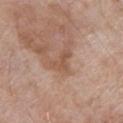Image and clinical context: A male patient in their 80s. From the chest. Imaged with white-light lighting. This image is a 15 mm lesion crop taken from a total-body photograph. Measured at roughly 3 mm in maximum diameter.